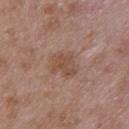Q: Was a biopsy performed?
A: imaged on a skin check; not biopsied
Q: What kind of image is this?
A: total-body-photography crop, ~15 mm field of view
Q: Lesion location?
A: the upper back
Q: How was the tile lit?
A: white-light illumination
Q: What are the patient's age and sex?
A: male, roughly 50 years of age
Q: How large is the lesion?
A: ~3.5 mm (longest diameter)